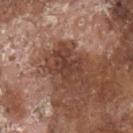size = ~6 mm (longest diameter) | location = the head or neck | automated lesion analysis = an area of roughly 14 mm²; a mean CIELAB color near L≈41 a*≈21 b*≈26, a lesion–skin lightness drop of about 10, and a normalized lesion–skin contrast near 8.5; a border-irregularity rating of about 4.5/10 and peripheral color asymmetry of about 1; an automated nevus-likeness rating near 0 out of 100 and a lesion-detection confidence of about 95/100 | patient = male, aged 78 to 82 | imaging modality = 15 mm crop, total-body photography.